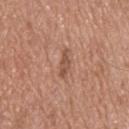No biopsy was performed on this lesion — it was imaged during a full skin examination and was not determined to be concerning.
Automated image analysis of the tile measured an area of roughly 3.5 mm² and a symmetry-axis asymmetry near 0.35. It also reported a mean CIELAB color near L≈52 a*≈22 b*≈29 and a normalized border contrast of about 6.5. It also reported an automated nevus-likeness rating near 0 out of 100 and a lesion-detection confidence of about 100/100.
The subject is a male in their mid- to late 50s.
The tile uses white-light illumination.
A region of skin cropped from a whole-body photographic capture, roughly 15 mm wide.
Longest diameter approximately 3.5 mm.
From the back.